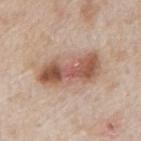Part of a total-body skin-imaging series; this lesion was reviewed on a skin check and was not flagged for biopsy. The lesion's longest dimension is about 7 mm. A male patient aged 63–67. From the mid back. A 15 mm close-up tile from a total-body photography series done for melanoma screening. Captured under white-light illumination.